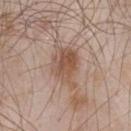Assessment: No biopsy was performed on this lesion — it was imaged during a full skin examination and was not determined to be concerning. Background: This image is a 15 mm lesion crop taken from a total-body photograph. The lesion-visualizer software estimated a footprint of about 12 mm², an eccentricity of roughly 0.75, and two-axis asymmetry of about 0.3. It also reported a border-irregularity index near 4/10, a color-variation rating of about 5.5/10, and radial color variation of about 2. The recorded lesion diameter is about 5 mm. From the chest. Imaged with white-light lighting. A male subject, aged approximately 55.